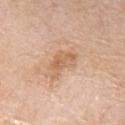Clinical impression: Part of a total-body skin-imaging series; this lesion was reviewed on a skin check and was not flagged for biopsy. Context: From the chest. Longest diameter approximately 4.5 mm. A male subject approximately 70 years of age. The total-body-photography lesion software estimated a border-irregularity rating of about 4.5/10 and a peripheral color-asymmetry measure near 1. Cropped from a total-body skin-imaging series; the visible field is about 15 mm.